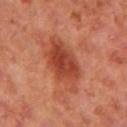The lesion was photographed on a routine skin check and not biopsied; there is no pathology result.
The subject is a female in their 40s.
The recorded lesion diameter is about 6.5 mm.
Captured under cross-polarized illumination.
A 15 mm crop from a total-body photograph taken for skin-cancer surveillance.
Located on the right upper arm.